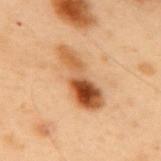The lesion was photographed on a routine skin check and not biopsied; there is no pathology result. The recorded lesion diameter is about 7 mm. A male patient roughly 55 years of age. This is a cross-polarized tile. Located on the mid back. A lesion tile, about 15 mm wide, cut from a 3D total-body photograph.